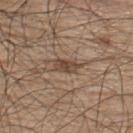Clinical impression:
This lesion was catalogued during total-body skin photography and was not selected for biopsy.
Image and clinical context:
The lesion is located on the upper back. Imaged with white-light lighting. A male subject, aged approximately 50. A 15 mm crop from a total-body photograph taken for skin-cancer surveillance. The lesion's longest dimension is about 3.5 mm.